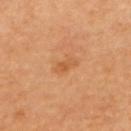- notes · imaged on a skin check; not biopsied
- anatomic site · the upper back
- TBP lesion metrics · a footprint of about 4 mm²; internal color variation of about 3 on a 0–10 scale and a peripheral color-asymmetry measure near 1
- acquisition · total-body-photography crop, ~15 mm field of view
- patient · female, aged 58–62